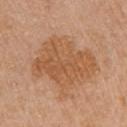Assessment: Imaged during a routine full-body skin examination; the lesion was not biopsied and no histopathology is available. Context: This is a white-light tile. From the left upper arm. A female subject in their mid- to late 50s. This image is a 15 mm lesion crop taken from a total-body photograph.